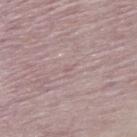follow-up: imaged on a skin check; not biopsied
image: 15 mm crop, total-body photography
size: ≈1 mm
subject: male, about 85 years old or older
automated metrics: an area of roughly 1 mm², a shape eccentricity near 0.8, and a shape-asymmetry score of about 0.4 (0 = symmetric); border irregularity of about 3 on a 0–10 scale, internal color variation of about 0 on a 0–10 scale, and radial color variation of about 0; a detector confidence of about 0 out of 100 that the crop contains a lesion
location: the upper back